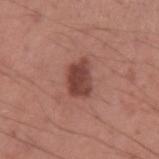This lesion was catalogued during total-body skin photography and was not selected for biopsy. This image is a 15 mm lesion crop taken from a total-body photograph. Automated image analysis of the tile measured a lesion color around L≈43 a*≈24 b*≈24 in CIELAB, about 13 CIELAB-L* units darker than the surrounding skin, and a normalized lesion–skin contrast near 9.5. From the arm. A male patient, about 35 years old. Captured under white-light illumination. Longest diameter approximately 4 mm.